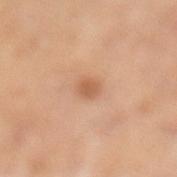biopsy status: imaged on a skin check; not biopsied | patient: female, aged 58–62 | image: 15 mm crop, total-body photography | anatomic site: the left lower leg.